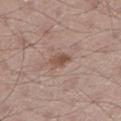biopsy status: total-body-photography surveillance lesion; no biopsy | location: the right lower leg | image: 15 mm crop, total-body photography | lesion diameter: about 2.5 mm | patient: male, aged approximately 35 | illumination: white-light | automated lesion analysis: an outline eccentricity of about 0.75 (0 = round, 1 = elongated) and a symmetry-axis asymmetry near 0.2; a color-variation rating of about 2.5/10.